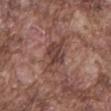workup: no biopsy performed (imaged during a skin exam)
automated metrics: an outline eccentricity of about 0.95 (0 = round, 1 = elongated) and two-axis asymmetry of about 0.3; an average lesion color of about L≈42 a*≈20 b*≈22 (CIELAB) and roughly 10 lightness units darker than nearby skin; a border-irregularity rating of about 5/10 and a within-lesion color-variation index near 3.5/10
location: the mid back
imaging modality: ~15 mm tile from a whole-body skin photo
patient: male, roughly 75 years of age
size: about 6.5 mm
lighting: white-light illumination